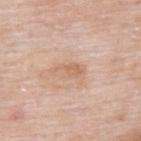<record>
<biopsy_status>not biopsied; imaged during a skin examination</biopsy_status>
</record>